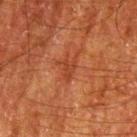<case>
  <biopsy_status>not biopsied; imaged during a skin examination</biopsy_status>
  <patient>
    <sex>male</sex>
    <age_approx>80</age_approx>
  </patient>
  <automated_metrics>
    <cielab_L>34</cielab_L>
    <cielab_a>25</cielab_a>
    <cielab_b>31</cielab_b>
    <vs_skin_darker_L>6.0</vs_skin_darker_L>
    <nevus_likeness_0_100>0</nevus_likeness_0_100>
    <lesion_detection_confidence_0_100>100</lesion_detection_confidence_0_100>
  </automated_metrics>
  <site>left upper arm</site>
  <lighting>cross-polarized</lighting>
  <image>
    <source>total-body photography crop</source>
    <field_of_view_mm>15</field_of_view_mm>
  </image>
  <lesion_size>
    <long_diameter_mm_approx>2.5</long_diameter_mm_approx>
  </lesion_size>
</case>